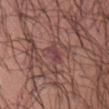A female patient, approximately 55 years of age.
Automated image analysis of the tile measured border irregularity of about 3 on a 0–10 scale. The analysis additionally found an automated nevus-likeness rating near 0 out of 100 and a lesion-detection confidence of about 85/100.
The lesion's longest dimension is about 2.5 mm.
On the abdomen.
A 15 mm close-up tile from a total-body photography series done for melanoma screening.